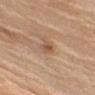biopsy_status: not biopsied; imaged during a skin examination
lighting: white-light
site: right upper arm
automated_metrics:
  eccentricity: 0.85
  shape_asymmetry: 0.3
image:
  source: total-body photography crop
  field_of_view_mm: 15
lesion_size:
  long_diameter_mm_approx: 3.0
patient:
  sex: male
  age_approx: 70